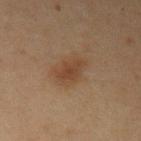<tbp_lesion>
<biopsy_status>not biopsied; imaged during a skin examination</biopsy_status>
<patient>
  <sex>female</sex>
  <age_approx>40</age_approx>
</patient>
<image>
  <source>total-body photography crop</source>
  <field_of_view_mm>15</field_of_view_mm>
</image>
<lighting>cross-polarized</lighting>
<automated_metrics>
  <cielab_L>35</cielab_L>
  <cielab_a>16</cielab_a>
  <cielab_b>27</cielab_b>
  <vs_skin_contrast_norm>6.5</vs_skin_contrast_norm>
  <border_irregularity_0_10>3.0</border_irregularity_0_10>
  <color_variation_0_10>2.5</color_variation_0_10>
  <peripheral_color_asymmetry>1.0</peripheral_color_asymmetry>
  <lesion_detection_confidence_0_100>100</lesion_detection_confidence_0_100>
</automated_metrics>
<site>left upper arm</site>
</tbp_lesion>